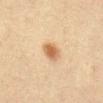The lesion was tiled from a total-body skin photograph and was not biopsied. A female patient, approximately 50 years of age. This image is a 15 mm lesion crop taken from a total-body photograph. The lesion is located on the abdomen.